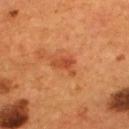<record>
<biopsy_status>not biopsied; imaged during a skin examination</biopsy_status>
<automated_metrics>
  <area_mm2_approx>4.0</area_mm2_approx>
  <eccentricity>0.8</eccentricity>
  <shape_asymmetry>0.55</shape_asymmetry>
  <cielab_L>45</cielab_L>
  <cielab_a>30</cielab_a>
  <cielab_b>38</cielab_b>
  <vs_skin_darker_L>9.0</vs_skin_darker_L>
  <vs_skin_contrast_norm>6.5</vs_skin_contrast_norm>
</automated_metrics>
<image>
  <source>total-body photography crop</source>
  <field_of_view_mm>15</field_of_view_mm>
</image>
<patient>
  <sex>male</sex>
  <age_approx>55</age_approx>
</patient>
<lighting>cross-polarized</lighting>
<site>upper back</site>
<lesion_size>
  <long_diameter_mm_approx>3.0</long_diameter_mm_approx>
</lesion_size>
</record>